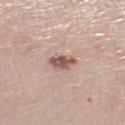  biopsy_status: not biopsied; imaged during a skin examination
  image:
    source: total-body photography crop
    field_of_view_mm: 15
  site: left lower leg
  lighting: white-light
  patient:
    sex: female
    age_approx: 55
  automated_metrics:
    area_mm2_approx: 6.0
    shape_asymmetry: 0.25
    cielab_L: 56
    cielab_a: 19
    cielab_b: 24
    vs_skin_darker_L: 13.0
    vs_skin_contrast_norm: 8.5
    lesion_detection_confidence_0_100: 100